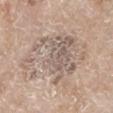{
  "biopsy_status": "not biopsied; imaged during a skin examination",
  "site": "left lower leg",
  "image": {
    "source": "total-body photography crop",
    "field_of_view_mm": 15
  },
  "lesion_size": {
    "long_diameter_mm_approx": 9.5
  },
  "lighting": "white-light",
  "patient": {
    "sex": "female",
    "age_approx": 75
  }
}